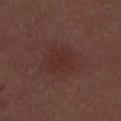The lesion was tiled from a total-body skin photograph and was not biopsied.
The patient is a male aged around 30.
Approximately 5 mm at its widest.
The total-body-photography lesion software estimated a lesion area of about 16 mm², an eccentricity of roughly 0.6, and two-axis asymmetry of about 0.25.
On the back.
This is a white-light tile.
Cropped from a whole-body photographic skin survey; the tile spans about 15 mm.